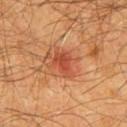| key | value |
|---|---|
| biopsy status | catalogued during a skin exam; not biopsied |
| acquisition | ~15 mm crop, total-body skin-cancer survey |
| body site | the front of the torso |
| patient | male, aged around 60 |